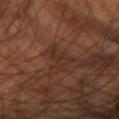<record>
  <biopsy_status>not biopsied; imaged during a skin examination</biopsy_status>
  <patient>
    <sex>male</sex>
    <age_approx>60</age_approx>
  </patient>
  <site>arm</site>
  <image>
    <source>total-body photography crop</source>
    <field_of_view_mm>15</field_of_view_mm>
  </image>
  <lesion_size>
    <long_diameter_mm_approx>3.5</long_diameter_mm_approx>
  </lesion_size>
</record>